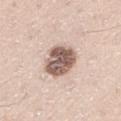<lesion>
  <biopsy_status>not biopsied; imaged during a skin examination</biopsy_status>
  <image>
    <source>total-body photography crop</source>
    <field_of_view_mm>15</field_of_view_mm>
  </image>
  <lesion_size>
    <long_diameter_mm_approx>4.0</long_diameter_mm_approx>
  </lesion_size>
  <lighting>white-light</lighting>
  <site>leg</site>
  <patient>
    <sex>male</sex>
    <age_approx>65</age_approx>
  </patient>
</lesion>